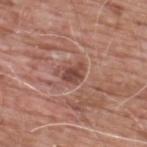{"biopsy_status": "not biopsied; imaged during a skin examination", "lighting": "white-light", "patient": {"sex": "male", "age_approx": 60}, "site": "upper back", "image": {"source": "total-body photography crop", "field_of_view_mm": 15}}